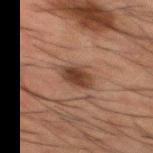{"biopsy_status": "not biopsied; imaged during a skin examination", "patient": {"sex": "male", "age_approx": 50}, "image": {"source": "total-body photography crop", "field_of_view_mm": 15}, "site": "mid back", "lighting": "cross-polarized"}